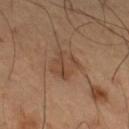Recorded during total-body skin imaging; not selected for excision or biopsy.
A lesion tile, about 15 mm wide, cut from a 3D total-body photograph.
Imaged with cross-polarized lighting.
The patient is a male aged approximately 65.
Located on the right thigh.
Longest diameter approximately 3.5 mm.